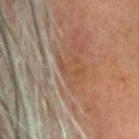The lesion is on the head or neck.
A male patient approximately 80 years of age.
Cropped from a whole-body photographic skin survey; the tile spans about 15 mm.
The recorded lesion diameter is about 3 mm.
The lesion-visualizer software estimated an area of roughly 3 mm² and a shape-asymmetry score of about 0.6 (0 = symmetric). The software also gave a nevus-likeness score of about 0/100 and a detector confidence of about 55 out of 100 that the crop contains a lesion.